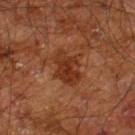This lesion was catalogued during total-body skin photography and was not selected for biopsy. A male subject aged 58–62. A roughly 15 mm field-of-view crop from a total-body skin photograph. The tile uses cross-polarized illumination. The lesion is located on the right leg. About 4.5 mm across.